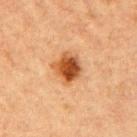Notes:
– notes · total-body-photography surveillance lesion; no biopsy
– subject · female, roughly 40 years of age
– acquisition · 15 mm crop, total-body photography
– body site · the right upper arm